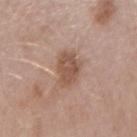The lesion was tiled from a total-body skin photograph and was not biopsied. A female subject, aged 43–47. The recorded lesion diameter is about 3.5 mm. A 15 mm close-up tile from a total-body photography series done for melanoma screening. On the left forearm.